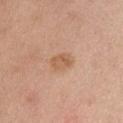The lesion is on the abdomen. Captured under white-light illumination. The subject is a female aged 63 to 67. A 15 mm crop from a total-body photograph taken for skin-cancer surveillance. Longest diameter approximately 2.5 mm.